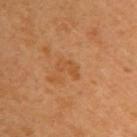No biopsy was performed on this lesion — it was imaged during a full skin examination and was not determined to be concerning.
The lesion is on the left arm.
The subject is a female aged around 45.
The lesion-visualizer software estimated a footprint of about 3.5 mm² and an outline eccentricity of about 0.85 (0 = round, 1 = elongated). The software also gave a mean CIELAB color near L≈51 a*≈24 b*≈40, about 6 CIELAB-L* units darker than the surrounding skin, and a normalized lesion–skin contrast near 5. And it measured a classifier nevus-likeness of about 0/100 and lesion-presence confidence of about 100/100.
Captured under cross-polarized illumination.
A 15 mm close-up extracted from a 3D total-body photography capture.